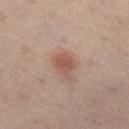biopsy status: no biopsy performed (imaged during a skin exam)
subject: female, roughly 55 years of age
image source: ~15 mm tile from a whole-body skin photo
site: the left thigh
size: ≈3 mm
illumination: cross-polarized illumination
TBP lesion metrics: an area of roughly 5.5 mm² and a shape-asymmetry score of about 0.35 (0 = symmetric)